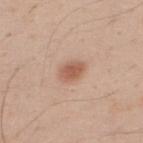Part of a total-body skin-imaging series; this lesion was reviewed on a skin check and was not flagged for biopsy.